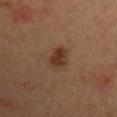  biopsy_status: not biopsied; imaged during a skin examination
  image:
    source: total-body photography crop
    field_of_view_mm: 15
  site: chest
  patient:
    sex: male
    age_approx: 35